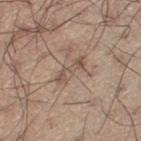Notes:
* biopsy status · imaged on a skin check; not biopsied
* imaging modality · ~15 mm crop, total-body skin-cancer survey
* lighting · white-light illumination
* subject · male, aged around 55
* automated lesion analysis · a footprint of about 5 mm², an outline eccentricity of about 0.95 (0 = round, 1 = elongated), and a symmetry-axis asymmetry near 0.25; a normalized border contrast of about 6; internal color variation of about 3 on a 0–10 scale and radial color variation of about 0.5
* anatomic site · the left thigh